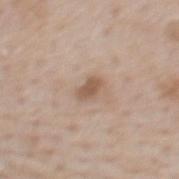This lesion was catalogued during total-body skin photography and was not selected for biopsy.
Located on the mid back.
A male patient in their mid- to late 60s.
The lesion's longest dimension is about 2.5 mm.
A 15 mm close-up extracted from a 3D total-body photography capture.
Captured under white-light illumination.
Automated tile analysis of the lesion measured a shape eccentricity near 0.75. The analysis additionally found a border-irregularity rating of about 2/10 and a within-lesion color-variation index near 1.5/10. It also reported lesion-presence confidence of about 100/100.